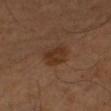biopsy status: catalogued during a skin exam; not biopsied
lighting: cross-polarized
patient: male, aged approximately 60
lesion size: ≈3 mm
image: ~15 mm tile from a whole-body skin photo
location: the arm
automated metrics: an area of roughly 6 mm², a shape eccentricity near 0.65, and a shape-asymmetry score of about 0.25 (0 = symmetric); a lesion color around L≈29 a*≈18 b*≈27 in CIELAB and a normalized lesion–skin contrast near 7.5